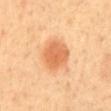The lesion is on the back. The patient is about 60 years old. The lesion's longest dimension is about 4 mm. The tile uses cross-polarized illumination. Cropped from a total-body skin-imaging series; the visible field is about 15 mm.